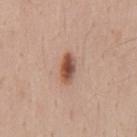This image is a 15 mm lesion crop taken from a total-body photograph.
Captured under white-light illumination.
The total-body-photography lesion software estimated an area of roughly 6 mm², an eccentricity of roughly 0.85, and a symmetry-axis asymmetry near 0.15. The software also gave an average lesion color of about L≈52 a*≈22 b*≈29 (CIELAB), roughly 15 lightness units darker than nearby skin, and a lesion-to-skin contrast of about 10 (normalized; higher = more distinct).
The lesion is on the mid back.
Approximately 3.5 mm at its widest.
A male subject about 35 years old.